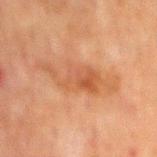notes — no biopsy performed (imaged during a skin exam); lesion size — ≈8 mm; lighting — cross-polarized illumination; site — the mid back; image — ~15 mm crop, total-body skin-cancer survey; patient — male, in their 60s.